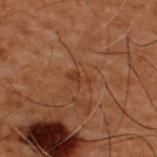biopsy status=imaged on a skin check; not biopsied | lesion diameter=≈2.5 mm | image=~15 mm tile from a whole-body skin photo | lighting=cross-polarized illumination | anatomic site=the upper back | subject=male, roughly 50 years of age.